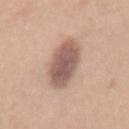The lesion was tiled from a total-body skin photograph and was not biopsied. A close-up tile cropped from a whole-body skin photograph, about 15 mm across. Longest diameter approximately 6.5 mm. From the mid back. The tile uses white-light illumination. A female subject roughly 40 years of age.